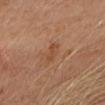– biopsy status — no biopsy performed (imaged during a skin exam)
– TBP lesion metrics — a footprint of about 3 mm², a shape eccentricity near 0.95, and a shape-asymmetry score of about 0.25 (0 = symmetric); a mean CIELAB color near L≈47 a*≈21 b*≈33 and a normalized border contrast of about 5.5
– illumination — cross-polarized illumination
– lesion diameter — ~3 mm (longest diameter)
– subject — female, in their 60s
– acquisition — 15 mm crop, total-body photography
– anatomic site — the head or neck